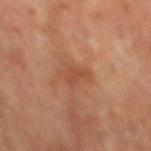The lesion was photographed on a routine skin check and not biopsied; there is no pathology result. Approximately 2.5 mm at its widest. Automated image analysis of the tile measured a lesion color around L≈35 a*≈20 b*≈26 in CIELAB, about 5 CIELAB-L* units darker than the surrounding skin, and a normalized lesion–skin contrast near 5. It also reported radial color variation of about 0.5. It also reported an automated nevus-likeness rating near 0 out of 100. On the mid back. Imaged with cross-polarized lighting. A male subject, about 60 years old. A 15 mm crop from a total-body photograph taken for skin-cancer surveillance.